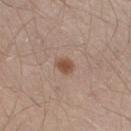Assessment:
Part of a total-body skin-imaging series; this lesion was reviewed on a skin check and was not flagged for biopsy.
Context:
The lesion is located on the right thigh. Approximately 2.5 mm at its widest. Captured under white-light illumination. A close-up tile cropped from a whole-body skin photograph, about 15 mm across. A male subject, aged 28 to 32.